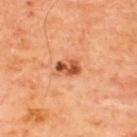* workup — total-body-photography surveillance lesion; no biopsy
* anatomic site — the upper back
* diameter — about 3 mm
* lighting — cross-polarized
* automated metrics — an outline eccentricity of about 0.75 (0 = round, 1 = elongated) and two-axis asymmetry of about 0.25; lesion-presence confidence of about 100/100
* subject — male, in their mid-60s
* image — ~15 mm tile from a whole-body skin photo